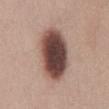Assessment: This lesion was catalogued during total-body skin photography and was not selected for biopsy. Context: Imaged with white-light lighting. A male subject approximately 25 years of age. A close-up tile cropped from a whole-body skin photograph, about 15 mm across. About 7 mm across. Automated image analysis of the tile measured an area of roughly 26 mm², an eccentricity of roughly 0.8, and a shape-asymmetry score of about 0.15 (0 = symmetric). And it measured a classifier nevus-likeness of about 90/100 and a lesion-detection confidence of about 100/100. The lesion is located on the chest.